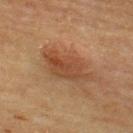biopsy_status: not biopsied; imaged during a skin examination
image:
  source: total-body photography crop
  field_of_view_mm: 15
automated_metrics:
  area_mm2_approx: 13.0
  eccentricity: 0.85
  cielab_L: 41
  cielab_a: 20
  cielab_b: 31
  vs_skin_darker_L: 9.0
patient:
  sex: male
  age_approx: 65
lighting: cross-polarized
site: upper back